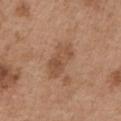subject = male, aged 53–57
illumination = white-light illumination
acquisition = ~15 mm crop, total-body skin-cancer survey
size = about 5 mm
anatomic site = the left upper arm